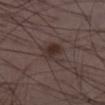{"lesion_size": {"long_diameter_mm_approx": 3.5}, "image": {"source": "total-body photography crop", "field_of_view_mm": 15}, "lighting": "white-light", "patient": {"sex": "male", "age_approx": 40}, "site": "leg", "automated_metrics": {"nevus_likeness_0_100": 95, "lesion_detection_confidence_0_100": 100}}